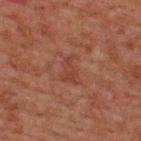Impression: Captured during whole-body skin photography for melanoma surveillance; the lesion was not biopsied. Background: Automated image analysis of the tile measured an area of roughly 5.5 mm². It also reported an average lesion color of about L≈31 a*≈19 b*≈23 (CIELAB), about 4 CIELAB-L* units darker than the surrounding skin, and a normalized lesion–skin contrast near 4.5. The software also gave a classifier nevus-likeness of about 0/100. The tile uses cross-polarized illumination. Longest diameter approximately 3.5 mm. A roughly 15 mm field-of-view crop from a total-body skin photograph. A male patient, roughly 60 years of age. On the upper back.